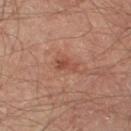{"biopsy_status": "not biopsied; imaged during a skin examination", "automated_metrics": {"color_variation_0_10": 0.5, "peripheral_color_asymmetry": 0.0, "nevus_likeness_0_100": 0, "lesion_detection_confidence_0_100": 100}, "site": "right thigh", "patient": {"sex": "male", "age_approx": 65}, "image": {"source": "total-body photography crop", "field_of_view_mm": 15}, "lesion_size": {"long_diameter_mm_approx": 2.5}}